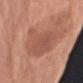| key | value |
|---|---|
| imaging modality | ~15 mm crop, total-body skin-cancer survey |
| site | the right upper arm |
| diameter | about 6.5 mm |
| patient | female, aged 73–77 |